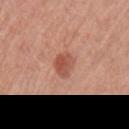No biopsy was performed on this lesion — it was imaged during a full skin examination and was not determined to be concerning.
A male subject, in their mid- to late 50s.
A region of skin cropped from a whole-body photographic capture, roughly 15 mm wide.
Longest diameter approximately 2.5 mm.
The lesion is on the left upper arm.
The tile uses white-light illumination.
Automated image analysis of the tile measured a footprint of about 5.5 mm², an eccentricity of roughly 0.35, and a symmetry-axis asymmetry near 0.2.